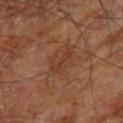Imaged during a routine full-body skin examination; the lesion was not biopsied and no histopathology is available.
A 15 mm close-up extracted from a 3D total-body photography capture.
The lesion is on the right leg.
The tile uses cross-polarized illumination.
The lesion's longest dimension is about 3 mm.
A male patient, aged around 60.
An algorithmic analysis of the crop reported a footprint of about 3 mm², an outline eccentricity of about 0.95 (0 = round, 1 = elongated), and two-axis asymmetry of about 0.3. And it measured a lesion color around L≈35 a*≈21 b*≈29 in CIELAB, a lesion–skin lightness drop of about 6, and a normalized lesion–skin contrast near 5.5.